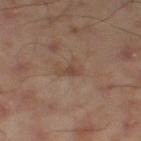workup: catalogued during a skin exam; not biopsied.